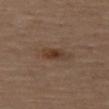Case summary:
• tile lighting — cross-polarized illumination
• image source — total-body-photography crop, ~15 mm field of view
• body site — the left thigh
• patient — male, aged approximately 65